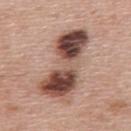Recorded during total-body skin imaging; not selected for excision or biopsy. A close-up tile cropped from a whole-body skin photograph, about 15 mm across. A male subject, in their 60s. The lesion is located on the upper back.